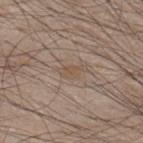Captured during whole-body skin photography for melanoma surveillance; the lesion was not biopsied. The lesion-visualizer software estimated a classifier nevus-likeness of about 0/100 and a lesion-detection confidence of about 80/100. Imaged with white-light lighting. The patient is a male aged around 45. A 15 mm crop from a total-body photograph taken for skin-cancer surveillance. Measured at roughly 3.5 mm in maximum diameter. The lesion is on the upper back.